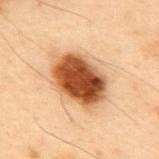Part of a total-body skin-imaging series; this lesion was reviewed on a skin check and was not flagged for biopsy.